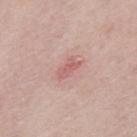biopsy_status: not biopsied; imaged during a skin examination
site: upper back
image:
  source: total-body photography crop
  field_of_view_mm: 15
patient:
  sex: male
  age_approx: 40